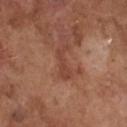Part of a total-body skin-imaging series; this lesion was reviewed on a skin check and was not flagged for biopsy.
The patient is a male in their mid-70s.
Imaged with white-light lighting.
About 4.5 mm across.
Cropped from a total-body skin-imaging series; the visible field is about 15 mm.
From the chest.